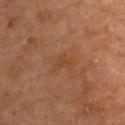biopsy_status: not biopsied; imaged during a skin examination
lighting: cross-polarized
image:
  source: total-body photography crop
  field_of_view_mm: 15
patient:
  sex: female
  age_approx: 70
lesion_size:
  long_diameter_mm_approx: 2.5
site: upper back
automated_metrics:
  area_mm2_approx: 3.0
  eccentricity: 0.8
  shape_asymmetry: 0.45
  nevus_likeness_0_100: 0
  lesion_detection_confidence_0_100: 100